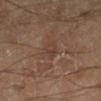Assessment:
This lesion was catalogued during total-body skin photography and was not selected for biopsy.
Acquisition and patient details:
From the right lower leg. Captured under cross-polarized illumination. Cropped from a total-body skin-imaging series; the visible field is about 15 mm. The subject is a male approximately 70 years of age. Measured at roughly 3 mm in maximum diameter.